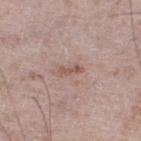Assessment: No biopsy was performed on this lesion — it was imaged during a full skin examination and was not determined to be concerning. Clinical summary: The lesion is on the left lower leg. A 15 mm close-up extracted from a 3D total-body photography capture. A male patient, approximately 75 years of age.